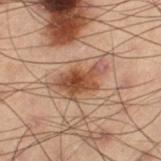workup = no biopsy performed (imaged during a skin exam)
anatomic site = the left thigh
subject = male, aged approximately 55
automated lesion analysis = a lesion color around L≈40 a*≈18 b*≈26 in CIELAB, a lesion–skin lightness drop of about 10, and a normalized lesion–skin contrast near 9; an automated nevus-likeness rating near 95 out of 100
lighting = cross-polarized
acquisition = total-body-photography crop, ~15 mm field of view